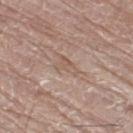| field | value |
|---|---|
| image | 15 mm crop, total-body photography |
| anatomic site | the left leg |
| size | about 3 mm |
| automated lesion analysis | an eccentricity of roughly 0.85; an average lesion color of about L≈56 a*≈17 b*≈26 (CIELAB); a within-lesion color-variation index near 1/10 and radial color variation of about 0; a classifier nevus-likeness of about 0/100 and a detector confidence of about 60 out of 100 that the crop contains a lesion |
| illumination | white-light illumination |
| patient | male, aged 78 to 82 |